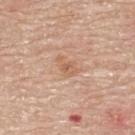The lesion was photographed on a routine skin check and not biopsied; there is no pathology result.
A male subject in their 70s.
Measured at roughly 3 mm in maximum diameter.
The lesion is on the upper back.
A 15 mm close-up tile from a total-body photography series done for melanoma screening.
The tile uses white-light illumination.